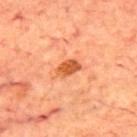workup: total-body-photography surveillance lesion; no biopsy | image source: ~15 mm crop, total-body skin-cancer survey | tile lighting: cross-polarized illumination | lesion size: about 3 mm | site: the upper back | subject: male, aged 68–72.